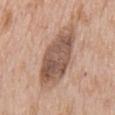Clinical impression: No biopsy was performed on this lesion — it was imaged during a full skin examination and was not determined to be concerning. Acquisition and patient details: The recorded lesion diameter is about 9 mm. The total-body-photography lesion software estimated an average lesion color of about L≈54 a*≈18 b*≈27 (CIELAB), a lesion–skin lightness drop of about 14, and a lesion-to-skin contrast of about 9 (normalized; higher = more distinct). The analysis additionally found a border-irregularity index near 2.5/10, internal color variation of about 5.5 on a 0–10 scale, and peripheral color asymmetry of about 2. The software also gave a nevus-likeness score of about 5/100 and a lesion-detection confidence of about 100/100. Located on the front of the torso. Imaged with white-light lighting. The patient is a male roughly 65 years of age. Cropped from a total-body skin-imaging series; the visible field is about 15 mm.